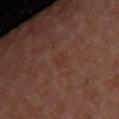biopsy status = no biopsy performed (imaged during a skin exam) | subject = female, in their mid- to late 40s | imaging modality = 15 mm crop, total-body photography | lesion diameter = ≈2 mm | site = the upper back | automated lesion analysis = a lesion area of about 2.5 mm², a shape eccentricity near 0.45, and a symmetry-axis asymmetry near 0.3; a nevus-likeness score of about 0/100 | lighting = cross-polarized illumination.